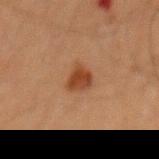biopsy status = no biopsy performed (imaged during a skin exam) | illumination = cross-polarized | acquisition = ~15 mm crop, total-body skin-cancer survey | subject = male, in their mid-60s | lesion diameter = ~3 mm (longest diameter) | site = the mid back.